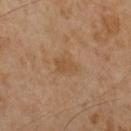A close-up tile cropped from a whole-body skin photograph, about 15 mm across. The tile uses cross-polarized illumination. A male patient, aged approximately 65. An algorithmic analysis of the crop reported a footprint of about 4 mm², a shape eccentricity near 0.75, and a symmetry-axis asymmetry near 0.3. It also reported border irregularity of about 2.5 on a 0–10 scale and peripheral color asymmetry of about 0.5. The analysis additionally found an automated nevus-likeness rating near 0 out of 100 and a detector confidence of about 100 out of 100 that the crop contains a lesion.